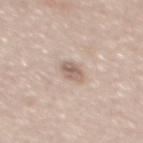No biopsy was performed on this lesion — it was imaged during a full skin examination and was not determined to be concerning.
An algorithmic analysis of the crop reported a shape eccentricity near 0.65. It also reported an average lesion color of about L≈61 a*≈15 b*≈24 (CIELAB), roughly 11 lightness units darker than nearby skin, and a lesion-to-skin contrast of about 7 (normalized; higher = more distinct). The software also gave a nevus-likeness score of about 10/100.
The patient is a male roughly 45 years of age.
The lesion's longest dimension is about 2.5 mm.
This image is a 15 mm lesion crop taken from a total-body photograph.
The tile uses white-light illumination.
The lesion is on the mid back.